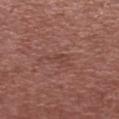workup — imaged on a skin check; not biopsied | tile lighting — white-light illumination | TBP lesion metrics — a mean CIELAB color near L≈43 a*≈23 b*≈25 and about 5 CIELAB-L* units darker than the surrounding skin; a border-irregularity index near 5.5/10, a color-variation rating of about 0.5/10, and a peripheral color-asymmetry measure near 0 | acquisition — 15 mm crop, total-body photography | patient — male, aged 53–57 | anatomic site — the left upper arm.